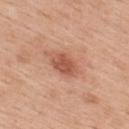Captured during whole-body skin photography for melanoma surveillance; the lesion was not biopsied. A roughly 15 mm field-of-view crop from a total-body skin photograph. Automated tile analysis of the lesion measured an area of roughly 7 mm², an eccentricity of roughly 0.8, and a symmetry-axis asymmetry near 0.15. The software also gave a lesion color around L≈55 a*≈26 b*≈32 in CIELAB, roughly 11 lightness units darker than nearby skin, and a normalized border contrast of about 7.5. It also reported a border-irregularity rating of about 1.5/10 and peripheral color asymmetry of about 1. A female subject, aged around 40. Measured at roughly 3.5 mm in maximum diameter. From the upper back. Captured under white-light illumination.